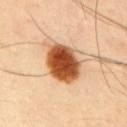biopsy_status: not biopsied; imaged during a skin examination
patient:
  sex: male
  age_approx: 50
image:
  source: total-body photography crop
  field_of_view_mm: 15
site: upper back